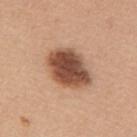follow-up: total-body-photography surveillance lesion; no biopsy | body site: the back | image: ~15 mm crop, total-body skin-cancer survey | image-analysis metrics: a lesion area of about 17 mm², an outline eccentricity of about 0.75 (0 = round, 1 = elongated), and two-axis asymmetry of about 0.15; an average lesion color of about L≈49 a*≈22 b*≈31 (CIELAB), a lesion–skin lightness drop of about 19, and a lesion-to-skin contrast of about 12.5 (normalized; higher = more distinct); border irregularity of about 1.5 on a 0–10 scale and peripheral color asymmetry of about 1.5 | subject: male, approximately 40 years of age | lighting: white-light illumination | size: about 5.5 mm.